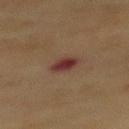Assessment: Captured during whole-body skin photography for melanoma surveillance; the lesion was not biopsied. Image and clinical context: From the mid back. Captured under cross-polarized illumination. Approximately 3 mm at its widest. A region of skin cropped from a whole-body photographic capture, roughly 15 mm wide. A female subject, aged 53–57.